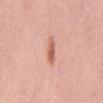| field | value |
|---|---|
| notes | imaged on a skin check; not biopsied |
| image source | 15 mm crop, total-body photography |
| subject | female, approximately 55 years of age |
| body site | the mid back |